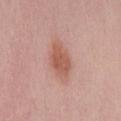Q: Is there a histopathology result?
A: total-body-photography surveillance lesion; no biopsy
Q: Who is the patient?
A: female, aged approximately 60
Q: How large is the lesion?
A: about 4.5 mm
Q: What is the imaging modality?
A: 15 mm crop, total-body photography
Q: Where on the body is the lesion?
A: the back